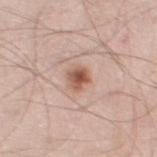follow-up = catalogued during a skin exam; not biopsied | image-analysis metrics = a lesion color around L≈56 a*≈21 b*≈29 in CIELAB and a normalized border contrast of about 9; a nevus-likeness score of about 95/100 and lesion-presence confidence of about 100/100 | acquisition = total-body-photography crop, ~15 mm field of view | subject = male, about 60 years old | anatomic site = the left thigh | lighting = white-light | diameter = ≈2.5 mm.